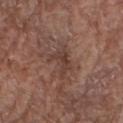<record>
<biopsy_status>not biopsied; imaged during a skin examination</biopsy_status>
<site>arm</site>
<lighting>white-light</lighting>
<image>
  <source>total-body photography crop</source>
  <field_of_view_mm>15</field_of_view_mm>
</image>
<automated_metrics>
  <area_mm2_approx>7.0</area_mm2_approx>
  <eccentricity>0.8</eccentricity>
  <shape_asymmetry>0.55</shape_asymmetry>
  <cielab_L>40</cielab_L>
  <cielab_a>19</cielab_a>
  <cielab_b>24</cielab_b>
  <vs_skin_darker_L>7.0</vs_skin_darker_L>
  <vs_skin_contrast_norm>6.5</vs_skin_contrast_norm>
</automated_metrics>
<patient>
  <sex>male</sex>
  <age_approx>70</age_approx>
</patient>
<lesion_size>
  <long_diameter_mm_approx>4.5</long_diameter_mm_approx>
</lesion_size>
</record>